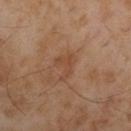image:
  source: total-body photography crop
  field_of_view_mm: 15
lesion_size:
  long_diameter_mm_approx: 3.5
patient:
  sex: male
  age_approx: 55
lighting: cross-polarized
automated_metrics:
  area_mm2_approx: 4.5
  eccentricity: 0.8
  shape_asymmetry: 0.65
  border_irregularity_0_10: 7.0
  color_variation_0_10: 1.5
  peripheral_color_asymmetry: 0.5
site: left upper arm